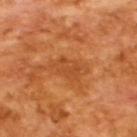<tbp_lesion>
  <biopsy_status>not biopsied; imaged during a skin examination</biopsy_status>
  <lighting>cross-polarized</lighting>
  <automated_metrics>
    <area_mm2_approx>6.0</area_mm2_approx>
    <eccentricity>0.65</eccentricity>
    <shape_asymmetry>0.25</shape_asymmetry>
    <vs_skin_darker_L>7.0</vs_skin_darker_L>
    <vs_skin_contrast_norm>5.0</vs_skin_contrast_norm>
    <border_irregularity_0_10>3.0</border_irregularity_0_10>
    <nevus_likeness_0_100>0</nevus_likeness_0_100>
  </automated_metrics>
  <image>
    <source>total-body photography crop</source>
    <field_of_view_mm>15</field_of_view_mm>
  </image>
  <patient>
    <sex>male</sex>
    <age_approx>65</age_approx>
  </patient>
</tbp_lesion>